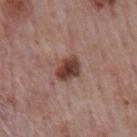Q: Is there a histopathology result?
A: no biopsy performed (imaged during a skin exam)
Q: How was this image acquired?
A: ~15 mm tile from a whole-body skin photo
Q: How was the tile lit?
A: white-light illumination
Q: Who is the patient?
A: male, approximately 75 years of age
Q: What is the lesion's diameter?
A: about 3 mm
Q: What is the anatomic site?
A: the back